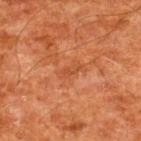Impression:
Imaged during a routine full-body skin examination; the lesion was not biopsied and no histopathology is available.
Acquisition and patient details:
A male subject, approximately 60 years of age. This is a cross-polarized tile. The lesion is located on the upper back. An algorithmic analysis of the crop reported a mean CIELAB color near L≈38 a*≈24 b*≈33, roughly 5 lightness units darker than nearby skin, and a normalized border contrast of about 4.5. It also reported a border-irregularity rating of about 4.5/10, a color-variation rating of about 0/10, and radial color variation of about 0. A 15 mm crop from a total-body photograph taken for skin-cancer surveillance. About 3 mm across.